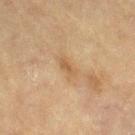Clinical impression: Recorded during total-body skin imaging; not selected for excision or biopsy. Image and clinical context: A female patient approximately 80 years of age. Cropped from a whole-body photographic skin survey; the tile spans about 15 mm. The lesion is on the leg.